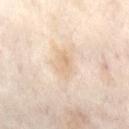A female patient in their mid-50s.
About 3.5 mm across.
A 15 mm crop from a total-body photograph taken for skin-cancer surveillance.
From the abdomen.
Captured under cross-polarized illumination.